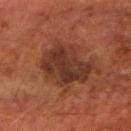body site: the right forearm | TBP lesion metrics: an area of roughly 20 mm², an outline eccentricity of about 0.8 (0 = round, 1 = elongated), and a shape-asymmetry score of about 0.25 (0 = symmetric); a lesion–skin lightness drop of about 9 and a lesion-to-skin contrast of about 8.5 (normalized; higher = more distinct); a border-irregularity rating of about 3/10, a within-lesion color-variation index near 3.5/10, and radial color variation of about 1; a classifier nevus-likeness of about 15/100 and a lesion-detection confidence of about 100/100 | lighting: cross-polarized illumination | imaging modality: 15 mm crop, total-body photography | patient: male, roughly 60 years of age.